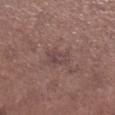Context:
A female subject, aged around 55. The tile uses white-light illumination. A 15 mm close-up tile from a total-body photography series done for melanoma screening. The lesion-visualizer software estimated a lesion color around L≈44 a*≈17 b*≈18 in CIELAB, a lesion–skin lightness drop of about 6, and a normalized border contrast of about 5.5. From the right lower leg.